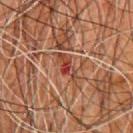Cropped from a whole-body photographic skin survey; the tile spans about 15 mm. Imaged with cross-polarized lighting. A male patient in their 80s. About 4 mm across. The lesion is on the chest.